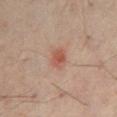The lesion was photographed on a routine skin check and not biopsied; there is no pathology result.
Captured under cross-polarized illumination.
Automated image analysis of the tile measured about 9 CIELAB-L* units darker than the surrounding skin and a lesion-to-skin contrast of about 7 (normalized; higher = more distinct). The analysis additionally found border irregularity of about 1.5 on a 0–10 scale and radial color variation of about 1.
About 2.5 mm across.
From the abdomen.
A lesion tile, about 15 mm wide, cut from a 3D total-body photograph.
The patient is a male roughly 60 years of age.